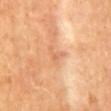Imaged during a routine full-body skin examination; the lesion was not biopsied and no histopathology is available.